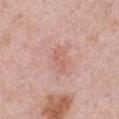  image:
    source: total-body photography crop
    field_of_view_mm: 15
  site: chest
  patient:
    sex: female
    age_approx: 50